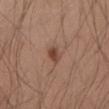Recorded during total-body skin imaging; not selected for excision or biopsy. Approximately 3 mm at its widest. This is a white-light tile. On the right thigh. A 15 mm crop from a total-body photograph taken for skin-cancer surveillance. Automated image analysis of the tile measured a footprint of about 3.5 mm² and a shape eccentricity near 0.85. The analysis additionally found a mean CIELAB color near L≈45 a*≈21 b*≈28. The software also gave a border-irregularity rating of about 2/10 and radial color variation of about 1. The subject is a male aged approximately 65.